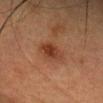The lesion was photographed on a routine skin check and not biopsied; there is no pathology result. Captured under cross-polarized illumination. The recorded lesion diameter is about 3.5 mm. A female patient aged 53 to 57. A 15 mm close-up extracted from a 3D total-body photography capture. The lesion is located on the head or neck.